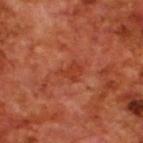No biopsy was performed on this lesion — it was imaged during a full skin examination and was not determined to be concerning.
A male subject, aged approximately 70.
Approximately 3 mm at its widest.
From the upper back.
Imaged with cross-polarized lighting.
Cropped from a whole-body photographic skin survey; the tile spans about 15 mm.